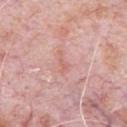Notes:
- notes · total-body-photography surveillance lesion; no biopsy
- imaging modality · ~15 mm crop, total-body skin-cancer survey
- patient · male, roughly 80 years of age
- anatomic site · the front of the torso
- lesion size · ≈3 mm